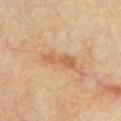Clinical impression:
Recorded during total-body skin imaging; not selected for excision or biopsy.
Background:
Imaged with cross-polarized lighting. A male patient, approximately 65 years of age. A region of skin cropped from a whole-body photographic capture, roughly 15 mm wide. Measured at roughly 4.5 mm in maximum diameter. The lesion is on the chest.